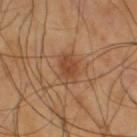Recorded during total-body skin imaging; not selected for excision or biopsy. About 3.5 mm across. A region of skin cropped from a whole-body photographic capture, roughly 15 mm wide. Located on the upper back. A male patient, roughly 40 years of age.